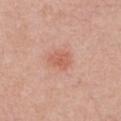Assessment:
The lesion was photographed on a routine skin check and not biopsied; there is no pathology result.
Background:
Cropped from a whole-body photographic skin survey; the tile spans about 15 mm. On the front of the torso. A female patient roughly 40 years of age. The recorded lesion diameter is about 3 mm. An algorithmic analysis of the crop reported an average lesion color of about L≈60 a*≈27 b*≈31 (CIELAB), a lesion–skin lightness drop of about 8, and a normalized lesion–skin contrast near 6. The analysis additionally found a border-irregularity rating of about 3/10 and peripheral color asymmetry of about 0.5. The software also gave lesion-presence confidence of about 100/100.